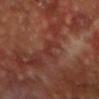Captured during whole-body skin photography for melanoma surveillance; the lesion was not biopsied. A close-up tile cropped from a whole-body skin photograph, about 15 mm across. Located on the head or neck. A male patient, approximately 55 years of age.